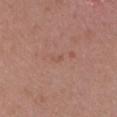The lesion was tiled from a total-body skin photograph and was not biopsied.